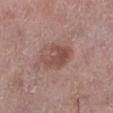No biopsy was performed on this lesion — it was imaged during a full skin examination and was not determined to be concerning.
A male patient in their mid- to late 50s.
The tile uses white-light illumination.
From the right lower leg.
Cropped from a whole-body photographic skin survey; the tile spans about 15 mm.